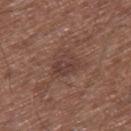workup: no biopsy performed (imaged during a skin exam) | diameter: ~3 mm (longest diameter) | illumination: white-light illumination | imaging modality: 15 mm crop, total-body photography | subject: male, approximately 75 years of age | body site: the right thigh.